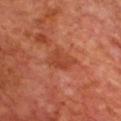Part of a total-body skin-imaging series; this lesion was reviewed on a skin check and was not flagged for biopsy. The lesion is located on the chest. A male subject, in their 70s. Automated image analysis of the tile measured a footprint of about 7.5 mm², a shape eccentricity near 0.75, and a symmetry-axis asymmetry near 0.3. And it measured a color-variation rating of about 2.5/10 and peripheral color asymmetry of about 1. A close-up tile cropped from a whole-body skin photograph, about 15 mm across. The recorded lesion diameter is about 4 mm. The tile uses cross-polarized illumination.